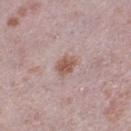| field | value |
|---|---|
| workup | imaged on a skin check; not biopsied |
| imaging modality | total-body-photography crop, ~15 mm field of view |
| subject | female, in their 40s |
| illumination | white-light illumination |
| site | the leg |